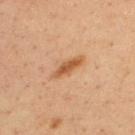The patient is a male about 35 years old. A region of skin cropped from a whole-body photographic capture, roughly 15 mm wide. Imaged with cross-polarized lighting. On the back.